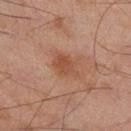Assessment:
The lesion was photographed on a routine skin check and not biopsied; there is no pathology result.
Background:
About 3.5 mm across. A 15 mm crop from a total-body photograph taken for skin-cancer surveillance. A male subject, about 45 years old. Imaged with cross-polarized lighting. Located on the leg. Automated image analysis of the tile measured a lesion area of about 6.5 mm² and a shape eccentricity near 0.7. The software also gave a lesion color around L≈39 a*≈19 b*≈26 in CIELAB, a lesion–skin lightness drop of about 7, and a lesion-to-skin contrast of about 6.5 (normalized; higher = more distinct). It also reported a color-variation rating of about 2/10 and radial color variation of about 1. It also reported an automated nevus-likeness rating near 15 out of 100 and a detector confidence of about 100 out of 100 that the crop contains a lesion.